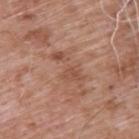* notes · total-body-photography surveillance lesion; no biopsy
* anatomic site · the back
* acquisition · 15 mm crop, total-body photography
* patient · male, about 60 years old
* tile lighting · white-light illumination
* image-analysis metrics · a lesion area of about 7.5 mm², a shape eccentricity near 0.9, and a symmetry-axis asymmetry near 0.5; a detector confidence of about 95 out of 100 that the crop contains a lesion